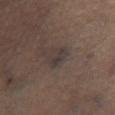Notes:
* TBP lesion metrics: an area of roughly 4 mm², a shape eccentricity near 0.8, and a shape-asymmetry score of about 0.25 (0 = symmetric); a lesion color around L≈33 a*≈10 b*≈15 in CIELAB and a lesion-to-skin contrast of about 7 (normalized; higher = more distinct); a classifier nevus-likeness of about 0/100 and lesion-presence confidence of about 65/100
* anatomic site: the left leg
* lighting: cross-polarized illumination
* lesion diameter: ≈3 mm
* patient: male, in their 50s
* image: ~15 mm crop, total-body skin-cancer survey